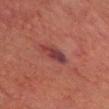Impression:
No biopsy was performed on this lesion — it was imaged during a full skin examination and was not determined to be concerning.
Clinical summary:
The lesion is on the head or neck. A male patient aged around 70. The recorded lesion diameter is about 3 mm. Cropped from a total-body skin-imaging series; the visible field is about 15 mm.